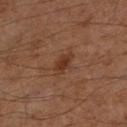  biopsy_status: not biopsied; imaged during a skin examination
  lesion_size:
    long_diameter_mm_approx: 3.0
  automated_metrics:
    cielab_L: 31
    cielab_a: 20
    cielab_b: 28
    vs_skin_darker_L: 7.0
    vs_skin_contrast_norm: 7.5
    border_irregularity_0_10: 3.0
    color_variation_0_10: 1.0
  site: left lower leg
  lighting: cross-polarized
  image:
    source: total-body photography crop
    field_of_view_mm: 15
  patient:
    sex: male
    age_approx: 60